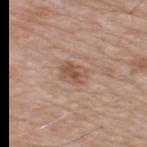  biopsy_status: not biopsied; imaged during a skin examination
  automated_metrics:
    cielab_L: 53
    cielab_a: 19
    cielab_b: 28
    vs_skin_darker_L: 9.0
    vs_skin_contrast_norm: 6.5
    border_irregularity_0_10: 2.0
    color_variation_0_10: 4.0
    peripheral_color_asymmetry: 1.5
  image:
    source: total-body photography crop
    field_of_view_mm: 15
  site: upper back
  patient:
    sex: male
    age_approx: 65